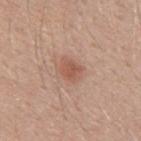The subject is a male in their 40s. Automated image analysis of the tile measured border irregularity of about 2.5 on a 0–10 scale, a color-variation rating of about 2/10, and a peripheral color-asymmetry measure near 0.5. This is a white-light tile. A 15 mm crop from a total-body photograph taken for skin-cancer surveillance. Located on the upper back. About 3 mm across.